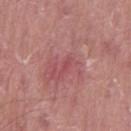Findings:
• biopsy status: imaged on a skin check; not biopsied
• image source: 15 mm crop, total-body photography
• lesion size: about 1.5 mm
• anatomic site: the front of the torso
• patient: male, aged 38–42
• image-analysis metrics: an outline eccentricity of about 0.85 (0 = round, 1 = elongated) and a symmetry-axis asymmetry near 0.4; border irregularity of about 3 on a 0–10 scale and a peripheral color-asymmetry measure near 0